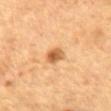workup: catalogued during a skin exam; not biopsied.